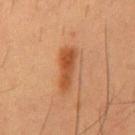Imaged during a routine full-body skin examination; the lesion was not biopsied and no histopathology is available. The subject is a male in their mid-50s. The tile uses cross-polarized illumination. The recorded lesion diameter is about 5.5 mm. On the mid back. The lesion-visualizer software estimated a shape eccentricity near 0.95 and a symmetry-axis asymmetry near 0.3. The analysis additionally found an average lesion color of about L≈44 a*≈24 b*≈35 (CIELAB), a lesion–skin lightness drop of about 10, and a normalized border contrast of about 8.5. It also reported a color-variation rating of about 3.5/10 and a peripheral color-asymmetry measure near 1. And it measured a classifier nevus-likeness of about 95/100 and a lesion-detection confidence of about 100/100. A 15 mm close-up tile from a total-body photography series done for melanoma screening.